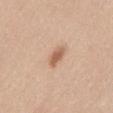Q: Was a biopsy performed?
A: total-body-photography surveillance lesion; no biopsy
Q: What is the imaging modality?
A: ~15 mm crop, total-body skin-cancer survey
Q: Where on the body is the lesion?
A: the mid back
Q: What lighting was used for the tile?
A: white-light illumination
Q: Patient demographics?
A: female, approximately 25 years of age
Q: What did automated image analysis measure?
A: a footprint of about 4 mm² and a shape-asymmetry score of about 0.25 (0 = symmetric); a mean CIELAB color near L≈60 a*≈21 b*≈32, about 12 CIELAB-L* units darker than the surrounding skin, and a lesion-to-skin contrast of about 7.5 (normalized; higher = more distinct); a border-irregularity index near 2.5/10 and a within-lesion color-variation index near 3/10; an automated nevus-likeness rating near 90 out of 100
Q: What is the lesion's diameter?
A: ~3 mm (longest diameter)